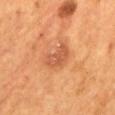This lesion was catalogued during total-body skin photography and was not selected for biopsy.
An algorithmic analysis of the crop reported an area of roughly 7.5 mm² and two-axis asymmetry of about 0.35.
This image is a 15 mm lesion crop taken from a total-body photograph.
Captured under cross-polarized illumination.
The lesion is located on the mid back.
A male subject, aged approximately 55.
About 4 mm across.